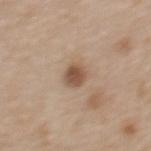workup=catalogued during a skin exam; not biopsied